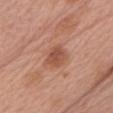<lesion>
<biopsy_status>not biopsied; imaged during a skin examination</biopsy_status>
</lesion>